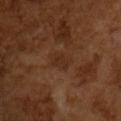Imaged during a routine full-body skin examination; the lesion was not biopsied and no histopathology is available. A male patient approximately 65 years of age. Imaged with cross-polarized lighting. Approximately 3.5 mm at its widest. An algorithmic analysis of the crop reported a mean CIELAB color near L≈28 a*≈18 b*≈28. A lesion tile, about 15 mm wide, cut from a 3D total-body photograph.